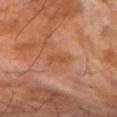<record>
<biopsy_status>not biopsied; imaged during a skin examination</biopsy_status>
<image>
  <source>total-body photography crop</source>
  <field_of_view_mm>15</field_of_view_mm>
</image>
<patient>
  <sex>male</sex>
  <age_approx>70</age_approx>
</patient>
<site>arm</site>
</record>